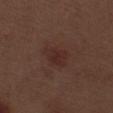Findings:
* biopsy status: total-body-photography surveillance lesion; no biopsy
* illumination: white-light
* diameter: ≈3.5 mm
* automated lesion analysis: an area of roughly 7.5 mm²; an average lesion color of about L≈28 a*≈18 b*≈21 (CIELAB), about 5 CIELAB-L* units darker than the surrounding skin, and a lesion-to-skin contrast of about 5.5 (normalized; higher = more distinct); a border-irregularity index near 2.5/10, a color-variation rating of about 2/10, and radial color variation of about 0.5; an automated nevus-likeness rating near 45 out of 100 and lesion-presence confidence of about 100/100
* image source: ~15 mm crop, total-body skin-cancer survey
* anatomic site: the left thigh
* subject: male, roughly 70 years of age